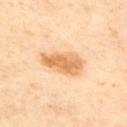No biopsy was performed on this lesion — it was imaged during a full skin examination and was not determined to be concerning. This is a cross-polarized tile. Approximately 5.5 mm at its widest. The lesion-visualizer software estimated an area of roughly 11 mm², a shape eccentricity near 0.9, and a shape-asymmetry score of about 0.25 (0 = symmetric). It also reported an automated nevus-likeness rating near 100 out of 100 and a lesion-detection confidence of about 100/100. A patient aged around 55. Located on the arm. A lesion tile, about 15 mm wide, cut from a 3D total-body photograph.